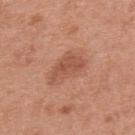workup=imaged on a skin check; not biopsied
lesion size=≈5 mm
automated lesion analysis=a detector confidence of about 100 out of 100 that the crop contains a lesion
anatomic site=the upper back
tile lighting=white-light illumination
patient=male, roughly 55 years of age
imaging modality=~15 mm crop, total-body skin-cancer survey